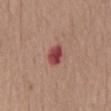The lesion was tiled from a total-body skin photograph and was not biopsied.
A male subject, about 75 years old.
A 15 mm close-up tile from a total-body photography series done for melanoma screening.
The lesion is on the chest.
Longest diameter approximately 3 mm.